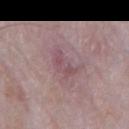notes: catalogued during a skin exam; not biopsied
anatomic site: the mid back
imaging modality: ~15 mm tile from a whole-body skin photo
lighting: white-light illumination
patient: male, approximately 65 years of age
automated metrics: a lesion area of about 6 mm², an eccentricity of roughly 0.85, and a shape-asymmetry score of about 0.4 (0 = symmetric); a mean CIELAB color near L≈51 a*≈21 b*≈14, a lesion–skin lightness drop of about 7, and a normalized border contrast of about 5.5; a border-irregularity index near 5/10, a within-lesion color-variation index near 2.5/10, and a peripheral color-asymmetry measure near 0.5; a nevus-likeness score of about 0/100
lesion diameter: ≈4 mm